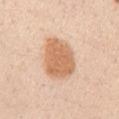{"biopsy_status": "not biopsied; imaged during a skin examination", "image": {"source": "total-body photography crop", "field_of_view_mm": 15}, "site": "right upper arm", "patient": {"sex": "female", "age_approx": 40}}